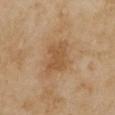Findings:
- follow-up — total-body-photography surveillance lesion; no biopsy
- lesion diameter — about 4 mm
- automated metrics — a nevus-likeness score of about 0/100
- illumination — cross-polarized illumination
- location — the chest
- patient — female, aged 33 to 37
- acquisition — ~15 mm tile from a whole-body skin photo